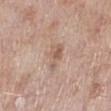Captured during whole-body skin photography for melanoma surveillance; the lesion was not biopsied. The subject is a female roughly 70 years of age. An algorithmic analysis of the crop reported an area of roughly 5.5 mm² and an eccentricity of roughly 0.85. It also reported border irregularity of about 4.5 on a 0–10 scale and a color-variation rating of about 3.5/10. It also reported a nevus-likeness score of about 0/100. The lesion is located on the leg. This image is a 15 mm lesion crop taken from a total-body photograph. The lesion's longest dimension is about 3.5 mm.